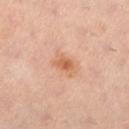Context: The tile uses cross-polarized illumination. Cropped from a whole-body photographic skin survey; the tile spans about 15 mm. The subject is a female aged 33–37. The lesion is located on the left lower leg. The recorded lesion diameter is about 3 mm.